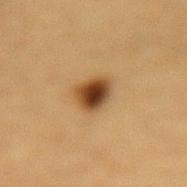The lesion was photographed on a routine skin check and not biopsied; there is no pathology result.
The subject is a male roughly 85 years of age or older.
A roughly 15 mm field-of-view crop from a total-body skin photograph.
About 3 mm across.
The tile uses cross-polarized illumination.
Located on the lower back.